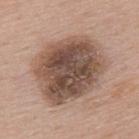Impression: Imaged during a routine full-body skin examination; the lesion was not biopsied and no histopathology is available.